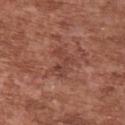| feature | finding |
|---|---|
| workup | total-body-photography surveillance lesion; no biopsy |
| subject | male, approximately 45 years of age |
| tile lighting | white-light illumination |
| site | the chest |
| image source | ~15 mm tile from a whole-body skin photo |
| image-analysis metrics | an outline eccentricity of about 0.7 (0 = round, 1 = elongated) and two-axis asymmetry of about 0.5; an average lesion color of about L≈43 a*≈24 b*≈27 (CIELAB); lesion-presence confidence of about 100/100 |
| lesion size | ≈4 mm |